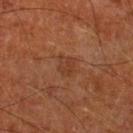<lesion>
<biopsy_status>not biopsied; imaged during a skin examination</biopsy_status>
<image>
  <source>total-body photography crop</source>
  <field_of_view_mm>15</field_of_view_mm>
</image>
<site>right lower leg</site>
<lesion_size>
  <long_diameter_mm_approx>2.5</long_diameter_mm_approx>
</lesion_size>
<automated_metrics>
  <area_mm2_approx>5.0</area_mm2_approx>
  <eccentricity>0.55</eccentricity>
  <shape_asymmetry>0.3</shape_asymmetry>
  <cielab_L>37</cielab_L>
  <cielab_a>23</cielab_a>
  <cielab_b>31</cielab_b>
  <vs_skin_darker_L>6.0</vs_skin_darker_L>
  <vs_skin_contrast_norm>5.0</vs_skin_contrast_norm>
  <border_irregularity_0_10>3.0</border_irregularity_0_10>
  <color_variation_0_10>2.0</color_variation_0_10>
  <nevus_likeness_0_100>0</nevus_likeness_0_100>
  <lesion_detection_confidence_0_100>100</lesion_detection_confidence_0_100>
</automated_metrics>
<patient>
  <sex>male</sex>
  <age_approx>65</age_approx>
</patient>
</lesion>